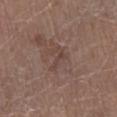Impression: Part of a total-body skin-imaging series; this lesion was reviewed on a skin check and was not flagged for biopsy. Background: A 15 mm close-up extracted from a 3D total-body photography capture. From the right lower leg. Approximately 3 mm at its widest. A female patient, aged 78–82. Imaged with white-light lighting.